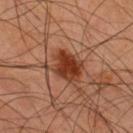Q: Is there a histopathology result?
A: imaged on a skin check; not biopsied
Q: Patient demographics?
A: male, aged approximately 45
Q: What is the imaging modality?
A: ~15 mm crop, total-body skin-cancer survey
Q: Lesion size?
A: about 4.5 mm
Q: How was the tile lit?
A: cross-polarized illumination
Q: Lesion location?
A: the upper back